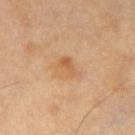biopsy_status: not biopsied; imaged during a skin examination
patient:
  sex: female
  age_approx: 60
lesion_size:
  long_diameter_mm_approx: 3.0
image:
  source: total-body photography crop
  field_of_view_mm: 15
site: leg
automated_metrics:
  area_mm2_approx: 4.0
  cielab_L: 61
  cielab_a: 22
  cielab_b: 40
  vs_skin_darker_L: 8.0
  vs_skin_contrast_norm: 6.0
  color_variation_0_10: 2.5
  peripheral_color_asymmetry: 1.0
  nevus_likeness_0_100: 10
  lesion_detection_confidence_0_100: 100